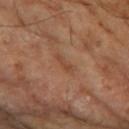Findings:
– notes · imaged on a skin check; not biopsied
– image-analysis metrics · a mean CIELAB color near L≈44 a*≈21 b*≈31, roughly 6 lightness units darker than nearby skin, and a normalized border contrast of about 5.5; an automated nevus-likeness rating near 0 out of 100 and a detector confidence of about 100 out of 100 that the crop contains a lesion
– image · 15 mm crop, total-body photography
– illumination · cross-polarized
– lesion size · ≈3 mm
– patient · male, in their mid- to late 60s